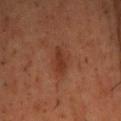Captured during whole-body skin photography for melanoma surveillance; the lesion was not biopsied.
Automated image analysis of the tile measured a footprint of about 4.5 mm², an outline eccentricity of about 0.95 (0 = round, 1 = elongated), and two-axis asymmetry of about 0.35. The analysis additionally found an average lesion color of about L≈31 a*≈22 b*≈28 (CIELAB), about 7 CIELAB-L* units darker than the surrounding skin, and a normalized border contrast of about 7. The analysis additionally found a classifier nevus-likeness of about 45/100 and a detector confidence of about 95 out of 100 that the crop contains a lesion.
A male subject, roughly 50 years of age.
About 4 mm across.
The lesion is on the head or neck.
Captured under cross-polarized illumination.
A 15 mm close-up extracted from a 3D total-body photography capture.